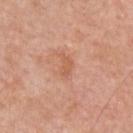Q: Is there a histopathology result?
A: no biopsy performed (imaged during a skin exam)
Q: Lesion size?
A: ~2.5 mm (longest diameter)
Q: What is the anatomic site?
A: the chest
Q: What is the imaging modality?
A: 15 mm crop, total-body photography
Q: What are the patient's age and sex?
A: male, aged around 50
Q: Automated lesion metrics?
A: a border-irregularity index near 4/10, a within-lesion color-variation index near 0.5/10, and radial color variation of about 0; an automated nevus-likeness rating near 0 out of 100 and a detector confidence of about 100 out of 100 that the crop contains a lesion
Q: Illumination type?
A: white-light illumination